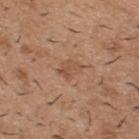Q: Is there a histopathology result?
A: catalogued during a skin exam; not biopsied
Q: Where on the body is the lesion?
A: the upper back
Q: What is the imaging modality?
A: ~15 mm tile from a whole-body skin photo
Q: What lighting was used for the tile?
A: white-light
Q: What are the patient's age and sex?
A: male, aged 38–42
Q: Lesion size?
A: about 3 mm